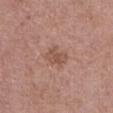Acquisition and patient details: Cropped from a whole-body photographic skin survey; the tile spans about 15 mm. The tile uses white-light illumination. An algorithmic analysis of the crop reported an area of roughly 5.5 mm², an eccentricity of roughly 0.7, and a symmetry-axis asymmetry near 0.35. The analysis additionally found an average lesion color of about L≈52 a*≈20 b*≈27 (CIELAB). And it measured border irregularity of about 3.5 on a 0–10 scale, a color-variation rating of about 2/10, and a peripheral color-asymmetry measure near 0.5. The software also gave an automated nevus-likeness rating near 0 out of 100 and a lesion-detection confidence of about 100/100. The lesion is on the abdomen. A female patient aged 63–67.